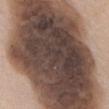workup — total-body-photography surveillance lesion; no biopsy
imaging modality — ~15 mm tile from a whole-body skin photo
subject — female, aged 63 to 67
size — ≈22 mm
illumination — white-light
automated metrics — a footprint of about 195 mm², an eccentricity of roughly 0.85, and a shape-asymmetry score of about 0.15 (0 = symmetric)
body site — the abdomen